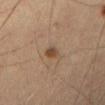Impression: Imaged during a routine full-body skin examination; the lesion was not biopsied and no histopathology is available. Background: A roughly 15 mm field-of-view crop from a total-body skin photograph. Imaged with cross-polarized lighting. About 2 mm across. The patient is a male roughly 70 years of age. From the right thigh. An algorithmic analysis of the crop reported an area of roughly 3 mm², a shape eccentricity near 0.6, and a symmetry-axis asymmetry near 0.3.